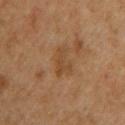A 15 mm close-up tile from a total-body photography series done for melanoma screening.
This is a cross-polarized tile.
The patient is a male about 60 years old.
Located on the upper back.
Approximately 4 mm at its widest.